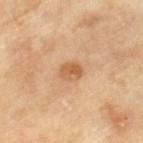Impression:
Recorded during total-body skin imaging; not selected for excision or biopsy.
Clinical summary:
Cropped from a whole-body photographic skin survey; the tile spans about 15 mm. The lesion-visualizer software estimated an eccentricity of roughly 0.7. It also reported a lesion-detection confidence of about 100/100. A male subject, approximately 65 years of age.